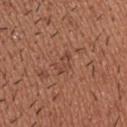The lesion was tiled from a total-body skin photograph and was not biopsied. This is a white-light tile. A 15 mm crop from a total-body photograph taken for skin-cancer surveillance. An algorithmic analysis of the crop reported an average lesion color of about L≈45 a*≈23 b*≈28 (CIELAB), about 6 CIELAB-L* units darker than the surrounding skin, and a normalized lesion–skin contrast near 5. It also reported a nevus-likeness score of about 0/100 and a lesion-detection confidence of about 90/100. The subject is a male in their 40s. On the upper back. The lesion's longest dimension is about 3 mm.